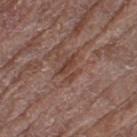The lesion was tiled from a total-body skin photograph and was not biopsied.
The tile uses white-light illumination.
A close-up tile cropped from a whole-body skin photograph, about 15 mm across.
From the left thigh.
A female patient aged around 80.